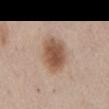Q: Was a biopsy performed?
A: imaged on a skin check; not biopsied
Q: What are the patient's age and sex?
A: male, aged 58–62
Q: What is the imaging modality?
A: 15 mm crop, total-body photography
Q: What is the anatomic site?
A: the abdomen